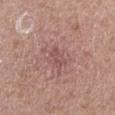| field | value |
|---|---|
| acquisition | ~15 mm tile from a whole-body skin photo |
| subject | male, in their 50s |
| site | the leg |
| size | ~2.5 mm (longest diameter) |
| TBP lesion metrics | an outline eccentricity of about 0.85 (0 = round, 1 = elongated) and a shape-asymmetry score of about 0.3 (0 = symmetric); a lesion color around L≈51 a*≈23 b*≈22 in CIELAB, a lesion–skin lightness drop of about 7, and a lesion-to-skin contrast of about 5 (normalized; higher = more distinct); a within-lesion color-variation index near 1.5/10 |